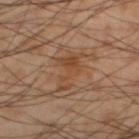{"biopsy_status": "not biopsied; imaged during a skin examination", "lesion_size": {"long_diameter_mm_approx": 5.0}, "automated_metrics": {"border_irregularity_0_10": 8.0, "color_variation_0_10": 3.0, "peripheral_color_asymmetry": 1.0, "nevus_likeness_0_100": 0, "lesion_detection_confidence_0_100": 100}, "image": {"source": "total-body photography crop", "field_of_view_mm": 15}, "site": "left lower leg", "patient": {"sex": "male", "age_approx": 55}}